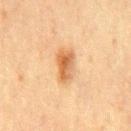Imaged during a routine full-body skin examination; the lesion was not biopsied and no histopathology is available. A close-up tile cropped from a whole-body skin photograph, about 15 mm across. The lesion is located on the mid back. This is a cross-polarized tile. The lesion's longest dimension is about 3.5 mm. A male subject, aged around 70.